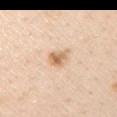Q: Patient demographics?
A: male, roughly 60 years of age
Q: What is the anatomic site?
A: the left upper arm
Q: What is the lesion's diameter?
A: ≈3 mm
Q: What lighting was used for the tile?
A: white-light illumination
Q: How was this image acquired?
A: ~15 mm crop, total-body skin-cancer survey